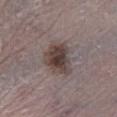The lesion was tiled from a total-body skin photograph and was not biopsied. A male subject, aged approximately 65. About 4 mm across. The lesion is located on the left lower leg. A roughly 15 mm field-of-view crop from a total-body skin photograph.